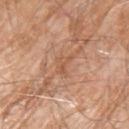Part of a total-body skin-imaging series; this lesion was reviewed on a skin check and was not flagged for biopsy.
A male subject aged 78 to 82.
On the left upper arm.
A roughly 15 mm field-of-view crop from a total-body skin photograph.
Approximately 2.5 mm at its widest.
The total-body-photography lesion software estimated a lesion color around L≈56 a*≈22 b*≈34 in CIELAB and roughly 7 lightness units darker than nearby skin. The software also gave a border-irregularity index near 5.5/10 and peripheral color asymmetry of about 0.